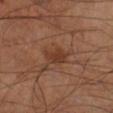| feature | finding |
|---|---|
| workup | total-body-photography surveillance lesion; no biopsy |
| subject | male, in their mid-60s |
| acquisition | ~15 mm crop, total-body skin-cancer survey |
| location | the left lower leg |
| illumination | cross-polarized illumination |